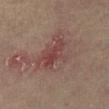Part of a total-body skin-imaging series; this lesion was reviewed on a skin check and was not flagged for biopsy. A female patient, approximately 80 years of age. About 6 mm across. This image is a 15 mm lesion crop taken from a total-body photograph. Imaged with cross-polarized lighting. The lesion is on the right lower leg.